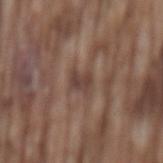workup = no biopsy performed (imaged during a skin exam)
image-analysis metrics = a lesion area of about 4.5 mm², an eccentricity of roughly 0.75, and two-axis asymmetry of about 0.35; an average lesion color of about L≈41 a*≈16 b*≈22 (CIELAB), about 8 CIELAB-L* units darker than the surrounding skin, and a normalized lesion–skin contrast near 7; lesion-presence confidence of about 60/100
image source = ~15 mm crop, total-body skin-cancer survey
lesion size = ≈3 mm
location = the mid back
patient = male, aged around 75
illumination = white-light